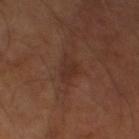Case summary:
• lesion diameter · about 3.5 mm
• subject · male, aged 63–67
• illumination · cross-polarized
• acquisition · total-body-photography crop, ~15 mm field of view